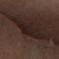This lesion was catalogued during total-body skin photography and was not selected for biopsy. Approximately 7 mm at its widest. This is a cross-polarized tile. On the right forearm. A male subject, aged approximately 60. Automated tile analysis of the lesion measured a footprint of about 22 mm² and a symmetry-axis asymmetry near 0.25. The analysis additionally found a mean CIELAB color near L≈17 a*≈11 b*≈14 and a lesion-to-skin contrast of about 7 (normalized; higher = more distinct). The software also gave a border-irregularity index near 5/10, a within-lesion color-variation index near 3/10, and a peripheral color-asymmetry measure near 1. This image is a 15 mm lesion crop taken from a total-body photograph.